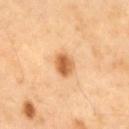The lesion was tiled from a total-body skin photograph and was not biopsied. The subject is a male aged around 40. A lesion tile, about 15 mm wide, cut from a 3D total-body photograph. On the mid back. Approximately 3 mm at its widest. The lesion-visualizer software estimated a mean CIELAB color near L≈63 a*≈25 b*≈43, a lesion–skin lightness drop of about 15, and a normalized border contrast of about 9. The tile uses cross-polarized illumination.